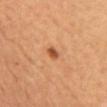The lesion was photographed on a routine skin check and not biopsied; there is no pathology result. A lesion tile, about 15 mm wide, cut from a 3D total-body photograph. The lesion-visualizer software estimated a border-irregularity rating of about 2.5/10 and peripheral color asymmetry of about 1. The analysis additionally found lesion-presence confidence of about 100/100. A female patient. From the front of the torso.